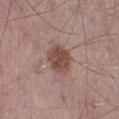Case summary:
* notes · catalogued during a skin exam; not biopsied
* automated metrics · a lesion area of about 8.5 mm², an outline eccentricity of about 0.65 (0 = round, 1 = elongated), and two-axis asymmetry of about 0.15; a border-irregularity rating of about 1/10, a color-variation rating of about 3.5/10, and radial color variation of about 1.5; a nevus-likeness score of about 55/100 and a detector confidence of about 100 out of 100 that the crop contains a lesion
* patient · male, about 65 years old
* size · about 3.5 mm
* imaging modality · total-body-photography crop, ~15 mm field of view
* body site · the leg